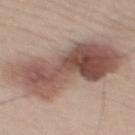This lesion was catalogued during total-body skin photography and was not selected for biopsy. The recorded lesion diameter is about 11 mm. The total-body-photography lesion software estimated an average lesion color of about L≈52 a*≈19 b*≈23 (CIELAB), roughly 14 lightness units darker than nearby skin, and a normalized lesion–skin contrast near 10. It also reported border irregularity of about 4.5 on a 0–10 scale and radial color variation of about 3.5. The software also gave a classifier nevus-likeness of about 95/100 and a detector confidence of about 100 out of 100 that the crop contains a lesion. This image is a 15 mm lesion crop taken from a total-body photograph. Located on the mid back. The tile uses white-light illumination. The patient is a male in their 40s.